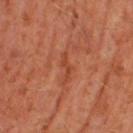On the right thigh. The tile uses cross-polarized illumination. This image is a 15 mm lesion crop taken from a total-body photograph. Longest diameter approximately 3 mm. A male patient aged 58–62.